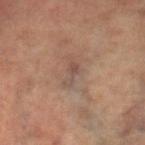Assessment: This lesion was catalogued during total-body skin photography and was not selected for biopsy. Background: The subject is a female aged around 65. This is a cross-polarized tile. A close-up tile cropped from a whole-body skin photograph, about 15 mm across. The lesion is on the leg. Approximately 3 mm at its widest.